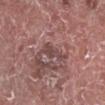<record>
<biopsy_status>not biopsied; imaged during a skin examination</biopsy_status>
<image>
  <source>total-body photography crop</source>
  <field_of_view_mm>15</field_of_view_mm>
</image>
<lighting>white-light</lighting>
<patient>
  <sex>male</sex>
  <age_approx>75</age_approx>
</patient>
<lesion_size>
  <long_diameter_mm_approx>4.0</long_diameter_mm_approx>
</lesion_size>
<automated_metrics>
  <area_mm2_approx>4.5</area_mm2_approx>
  <eccentricity>0.9</eccentricity>
  <shape_asymmetry>0.4</shape_asymmetry>
  <vs_skin_darker_L>9.0</vs_skin_darker_L>
  <vs_skin_contrast_norm>7.0</vs_skin_contrast_norm>
</automated_metrics>
<site>right lower leg</site>
</record>